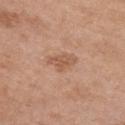The lesion was tiled from a total-body skin photograph and was not biopsied.
A female patient aged around 65.
From the chest.
A close-up tile cropped from a whole-body skin photograph, about 15 mm across.
Automated image analysis of the tile measured a lesion color around L≈56 a*≈22 b*≈31 in CIELAB, roughly 8 lightness units darker than nearby skin, and a normalized border contrast of about 6.
The tile uses white-light illumination.
The lesion's longest dimension is about 3.5 mm.